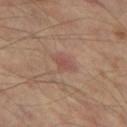Q: Was this lesion biopsied?
A: total-body-photography surveillance lesion; no biopsy
Q: What are the patient's age and sex?
A: male, approximately 55 years of age
Q: What kind of image is this?
A: ~15 mm tile from a whole-body skin photo
Q: Lesion location?
A: the left thigh